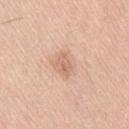Assessment:
Captured during whole-body skin photography for melanoma surveillance; the lesion was not biopsied.
Context:
Longest diameter approximately 3 mm. A 15 mm crop from a total-body photograph taken for skin-cancer surveillance. Imaged with white-light lighting. A male subject, aged approximately 35. From the lower back.